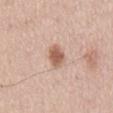biopsy status = imaged on a skin check; not biopsied
site = the mid back
illumination = white-light
acquisition = 15 mm crop, total-body photography
image-analysis metrics = a border-irregularity rating of about 2.5/10; an automated nevus-likeness rating near 95 out of 100 and a lesion-detection confidence of about 100/100
subject = male, in their mid- to late 40s
lesion diameter = ~3.5 mm (longest diameter)